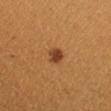Captured under cross-polarized illumination.
A female subject aged 28–32.
Approximately 2 mm at its widest.
Located on the arm.
This image is a 15 mm lesion crop taken from a total-body photograph.
The total-body-photography lesion software estimated an outline eccentricity of about 0.35 (0 = round, 1 = elongated) and two-axis asymmetry of about 0.25. The software also gave a border-irregularity index near 2/10, a color-variation rating of about 1.5/10, and a peripheral color-asymmetry measure near 0.5. And it measured a classifier nevus-likeness of about 100/100 and a detector confidence of about 100 out of 100 that the crop contains a lesion.